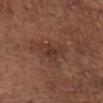Assessment:
No biopsy was performed on this lesion — it was imaged during a full skin examination and was not determined to be concerning.
Clinical summary:
An algorithmic analysis of the crop reported a lesion area of about 6 mm², a shape eccentricity near 0.7, and a shape-asymmetry score of about 0.55 (0 = symmetric). And it measured border irregularity of about 5.5 on a 0–10 scale, a within-lesion color-variation index near 2.5/10, and radial color variation of about 1. It also reported a classifier nevus-likeness of about 0/100 and a lesion-detection confidence of about 100/100. A male subject aged around 75. A 15 mm close-up tile from a total-body photography series done for melanoma screening. The lesion is on the chest. About 3.5 mm across. Captured under white-light illumination.